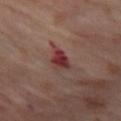This lesion was catalogued during total-body skin photography and was not selected for biopsy. The lesion is located on the left thigh. A region of skin cropped from a whole-body photographic capture, roughly 15 mm wide. Approximately 3.5 mm at its widest. The subject is a female roughly 55 years of age.